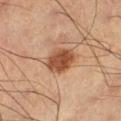No biopsy was performed on this lesion — it was imaged during a full skin examination and was not determined to be concerning.
This is a cross-polarized tile.
Automated image analysis of the tile measured a shape eccentricity near 0.6 and a symmetry-axis asymmetry near 0.15. It also reported an average lesion color of about L≈50 a*≈24 b*≈34 (CIELAB) and a lesion–skin lightness drop of about 14. The analysis additionally found a border-irregularity index near 1.5/10, a color-variation rating of about 4/10, and radial color variation of about 1.5. And it measured a classifier nevus-likeness of about 100/100.
A lesion tile, about 15 mm wide, cut from a 3D total-body photograph.
On the left lower leg.
The lesion's longest dimension is about 4 mm.
A male subject approximately 60 years of age.